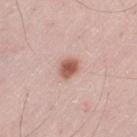follow-up — imaged on a skin check; not biopsied | image — ~15 mm tile from a whole-body skin photo | tile lighting — white-light illumination | size — about 2.5 mm | subject — male, aged 53–57 | anatomic site — the left thigh.